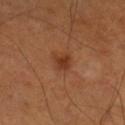Assessment: The lesion was photographed on a routine skin check and not biopsied; there is no pathology result. Clinical summary: A male patient in their mid- to late 50s. On the right lower leg. The tile uses cross-polarized illumination. About 2.5 mm across. A region of skin cropped from a whole-body photographic capture, roughly 15 mm wide.